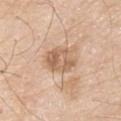No biopsy was performed on this lesion — it was imaged during a full skin examination and was not determined to be concerning. A male patient, approximately 80 years of age. A 15 mm crop from a total-body photograph taken for skin-cancer surveillance. The lesion is on the right upper arm.